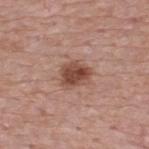patient: male, aged approximately 55
lesion size: ≈3.5 mm
image source: ~15 mm crop, total-body skin-cancer survey
image-analysis metrics: a lesion area of about 8 mm², an eccentricity of roughly 0.6, and a shape-asymmetry score of about 0.25 (0 = symmetric); a classifier nevus-likeness of about 95/100 and a lesion-detection confidence of about 100/100
anatomic site: the upper back
illumination: white-light illumination